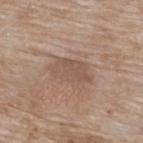Part of a total-body skin-imaging series; this lesion was reviewed on a skin check and was not flagged for biopsy.